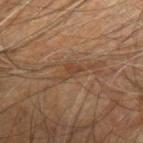Q: Was a biopsy performed?
A: catalogued during a skin exam; not biopsied
Q: Who is the patient?
A: male, aged 58–62
Q: Where on the body is the lesion?
A: the left arm
Q: What is the imaging modality?
A: 15 mm crop, total-body photography
Q: Automated lesion metrics?
A: a footprint of about 2.5 mm² and a shape eccentricity near 0.75; a mean CIELAB color near L≈40 a*≈18 b*≈30
Q: Lesion size?
A: ~2.5 mm (longest diameter)